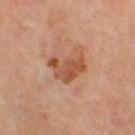Q: Is there a histopathology result?
A: no biopsy performed (imaged during a skin exam)
Q: Who is the patient?
A: female, aged 48–52
Q: How was this image acquired?
A: ~15 mm tile from a whole-body skin photo
Q: What is the anatomic site?
A: the right thigh
Q: What did automated image analysis measure?
A: an outline eccentricity of about 0.75 (0 = round, 1 = elongated); a mean CIELAB color near L≈54 a*≈25 b*≈35 and a normalized border contrast of about 8; a classifier nevus-likeness of about 10/100
Q: Lesion size?
A: about 4.5 mm
Q: How was the tile lit?
A: cross-polarized